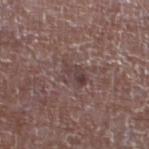Assessment: Captured during whole-body skin photography for melanoma surveillance; the lesion was not biopsied. Image and clinical context: From the left lower leg. A male subject aged approximately 65. A 15 mm crop from a total-body photograph taken for skin-cancer surveillance.